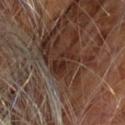workup — catalogued during a skin exam; not biopsied | imaging modality — 15 mm crop, total-body photography | body site — the chest | patient — male, about 60 years old.